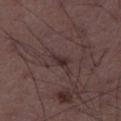biopsy status = no biopsy performed (imaged during a skin exam) | automated metrics = an area of roughly 3 mm², a shape eccentricity near 0.85, and two-axis asymmetry of about 0.35; an average lesion color of about L≈30 a*≈15 b*≈16 (CIELAB) and a normalized lesion–skin contrast near 7; lesion-presence confidence of about 90/100 | acquisition = total-body-photography crop, ~15 mm field of view | subject = male, in their 50s | size = ~2.5 mm (longest diameter) | body site = the right thigh.